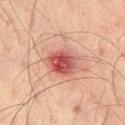{
  "biopsy_status": "not biopsied; imaged during a skin examination",
  "image": {
    "source": "total-body photography crop",
    "field_of_view_mm": 15
  },
  "site": "left thigh",
  "lighting": "cross-polarized",
  "lesion_size": {
    "long_diameter_mm_approx": 3.5
  },
  "patient": {
    "sex": "male",
    "age_approx": 35
  }
}